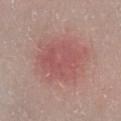The lesion was tiled from a total-body skin photograph and was not biopsied.